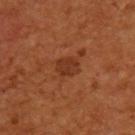Assessment: This lesion was catalogued during total-body skin photography and was not selected for biopsy.